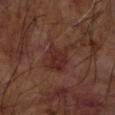biopsy status: total-body-photography surveillance lesion; no biopsy | body site: the left forearm | image source: ~15 mm tile from a whole-body skin photo | subject: male, about 65 years old | tile lighting: cross-polarized | automated lesion analysis: a footprint of about 11 mm², an outline eccentricity of about 0.75 (0 = round, 1 = elongated), and a shape-asymmetry score of about 0.45 (0 = symmetric); a nevus-likeness score of about 15/100.